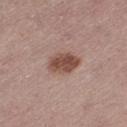workup: total-body-photography surveillance lesion; no biopsy
subject: female, approximately 55 years of age
site: the left thigh
tile lighting: white-light
image: total-body-photography crop, ~15 mm field of view